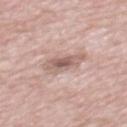Impression:
Imaged during a routine full-body skin examination; the lesion was not biopsied and no histopathology is available.
Image and clinical context:
An algorithmic analysis of the crop reported a lesion–skin lightness drop of about 11 and a normalized lesion–skin contrast near 7. This image is a 15 mm lesion crop taken from a total-body photograph. A male subject aged around 70. The lesion is located on the mid back. Imaged with white-light lighting. The recorded lesion diameter is about 4 mm.